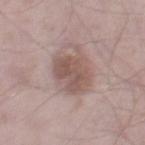<record>
  <site>right thigh</site>
  <patient>
    <sex>male</sex>
    <age_approx>55</age_approx>
  </patient>
  <image>
    <source>total-body photography crop</source>
    <field_of_view_mm>15</field_of_view_mm>
  </image>
</record>